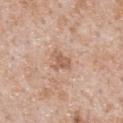{
  "image": {
    "source": "total-body photography crop",
    "field_of_view_mm": 15
  },
  "patient": {
    "sex": "male",
    "age_approx": 65
  },
  "site": "chest"
}